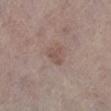Q: Was this lesion biopsied?
A: catalogued during a skin exam; not biopsied
Q: What is the imaging modality?
A: total-body-photography crop, ~15 mm field of view
Q: Automated lesion metrics?
A: roughly 7 lightness units darker than nearby skin and a normalized lesion–skin contrast near 5.5; internal color variation of about 1 on a 0–10 scale and a peripheral color-asymmetry measure near 0.5; a nevus-likeness score of about 10/100 and lesion-presence confidence of about 100/100
Q: What is the anatomic site?
A: the leg
Q: Patient demographics?
A: male, aged approximately 80
Q: How was the tile lit?
A: white-light
Q: Lesion size?
A: ≈2.5 mm